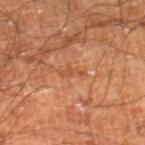workup: no biopsy performed (imaged during a skin exam) | size: about 2.5 mm | site: the leg | acquisition: total-body-photography crop, ~15 mm field of view | patient: male, aged around 60 | automated metrics: a nevus-likeness score of about 0/100 and a lesion-detection confidence of about 90/100 | tile lighting: cross-polarized illumination.